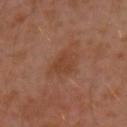Case summary:
- follow-up — no biopsy performed (imaged during a skin exam)
- patient — male, roughly 30 years of age
- tile lighting — cross-polarized
- TBP lesion metrics — a footprint of about 7.5 mm², a shape eccentricity near 0.75, and a symmetry-axis asymmetry near 0.3; a mean CIELAB color near L≈40 a*≈21 b*≈30 and a normalized lesion–skin contrast near 6; an automated nevus-likeness rating near 0 out of 100 and a detector confidence of about 100 out of 100 that the crop contains a lesion
- image source — ~15 mm crop, total-body skin-cancer survey
- site — the left upper arm